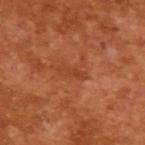Q: Was this lesion biopsied?
A: no biopsy performed (imaged during a skin exam)
Q: What is the imaging modality?
A: ~15 mm crop, total-body skin-cancer survey
Q: Illumination type?
A: cross-polarized
Q: What are the patient's age and sex?
A: male, roughly 65 years of age
Q: What did automated image analysis measure?
A: a footprint of about 3.5 mm² and a shape-asymmetry score of about 0.4 (0 = symmetric); an average lesion color of about L≈42 a*≈29 b*≈37 (CIELAB), roughly 6 lightness units darker than nearby skin, and a lesion-to-skin contrast of about 4.5 (normalized; higher = more distinct); a classifier nevus-likeness of about 0/100 and a lesion-detection confidence of about 100/100
Q: How large is the lesion?
A: ~3.5 mm (longest diameter)